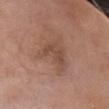{
  "biopsy_status": "not biopsied; imaged during a skin examination",
  "lighting": "white-light",
  "image": {
    "source": "total-body photography crop",
    "field_of_view_mm": 15
  },
  "site": "right upper arm",
  "patient": {
    "sex": "female",
    "age_approx": 75
  },
  "automated_metrics": {
    "area_mm2_approx": 8.5,
    "eccentricity": 0.65,
    "shape_asymmetry": 0.5,
    "border_irregularity_0_10": 6.5,
    "color_variation_0_10": 2.0,
    "peripheral_color_asymmetry": 0.5
  },
  "lesion_size": {
    "long_diameter_mm_approx": 4.0
  }
}